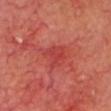Q: What lighting was used for the tile?
A: cross-polarized
Q: How was this image acquired?
A: total-body-photography crop, ~15 mm field of view
Q: Patient demographics?
A: male, aged approximately 65
Q: What is the anatomic site?
A: the head or neck
Q: How large is the lesion?
A: ~3 mm (longest diameter)